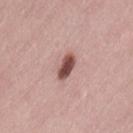workup: total-body-photography surveillance lesion; no biopsy | site: the back | patient: female, in their 40s | imaging modality: total-body-photography crop, ~15 mm field of view | illumination: white-light | lesion size: about 3 mm.